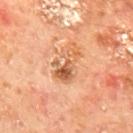Impression: The lesion was tiled from a total-body skin photograph and was not biopsied. Context: Cropped from a whole-body photographic skin survey; the tile spans about 15 mm. Located on the mid back. A male subject, in their mid- to late 60s. Measured at roughly 4.5 mm in maximum diameter. Imaged with cross-polarized lighting.